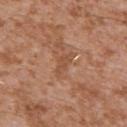Impression:
The lesion was photographed on a routine skin check and not biopsied; there is no pathology result.
Context:
This is a white-light tile. From the left upper arm. A male subject approximately 45 years of age. This image is a 15 mm lesion crop taken from a total-body photograph. Measured at roughly 3.5 mm in maximum diameter. The lesion-visualizer software estimated a footprint of about 4 mm², an outline eccentricity of about 0.9 (0 = round, 1 = elongated), and a shape-asymmetry score of about 0.4 (0 = symmetric). It also reported an average lesion color of about L≈51 a*≈22 b*≈33 (CIELAB), roughly 7 lightness units darker than nearby skin, and a lesion-to-skin contrast of about 5 (normalized; higher = more distinct). And it measured border irregularity of about 4.5 on a 0–10 scale, a within-lesion color-variation index near 1/10, and a peripheral color-asymmetry measure near 0.5. It also reported a nevus-likeness score of about 0/100 and a lesion-detection confidence of about 85/100.